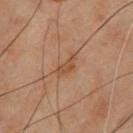notes: total-body-photography surveillance lesion; no biopsy
lighting: cross-polarized
patient: male, aged 53–57
lesion diameter: ≈3.5 mm
image: ~15 mm tile from a whole-body skin photo
site: the chest